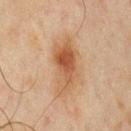  biopsy_status: not biopsied; imaged during a skin examination
  lighting: cross-polarized
  site: chest
  image:
    source: total-body photography crop
    field_of_view_mm: 15
  patient:
    sex: male
    age_approx: 45
  lesion_size:
    long_diameter_mm_approx: 6.5